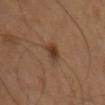Impression:
No biopsy was performed on this lesion — it was imaged during a full skin examination and was not determined to be concerning.
Image and clinical context:
A male patient, aged 48 to 52. From the left upper arm. A 15 mm close-up extracted from a 3D total-body photography capture.